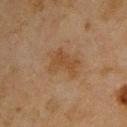Findings:
• subject: male, aged approximately 45
• site: the upper back
• lesion size: ~4.5 mm (longest diameter)
• acquisition: total-body-photography crop, ~15 mm field of view